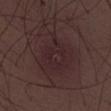Q: Was a biopsy performed?
A: no biopsy performed (imaged during a skin exam)
Q: How large is the lesion?
A: about 8 mm
Q: How was the tile lit?
A: white-light illumination
Q: Who is the patient?
A: male, approximately 50 years of age
Q: What is the imaging modality?
A: ~15 mm tile from a whole-body skin photo